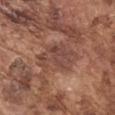Captured during whole-body skin photography for melanoma surveillance; the lesion was not biopsied.